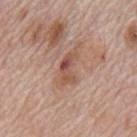notes: imaged on a skin check; not biopsied | diameter: ≈4.5 mm | location: the mid back | subject: male, roughly 70 years of age | image source: ~15 mm tile from a whole-body skin photo.